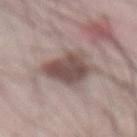Assessment:
Part of a total-body skin-imaging series; this lesion was reviewed on a skin check and was not flagged for biopsy.
Acquisition and patient details:
Approximately 5.5 mm at its widest. The patient is a male aged 68–72. The lesion is located on the abdomen. A 15 mm crop from a total-body photograph taken for skin-cancer surveillance. The tile uses white-light illumination.